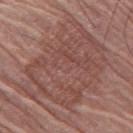biopsy status = catalogued during a skin exam; not biopsied | location = the left thigh | image = ~15 mm crop, total-body skin-cancer survey | subject = female, aged 78–82 | size = about 10.5 mm.